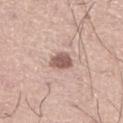<record>
<biopsy_status>not biopsied; imaged during a skin examination</biopsy_status>
<automated_metrics>
  <area_mm2_approx>5.0</area_mm2_approx>
  <eccentricity>0.45</eccentricity>
  <shape_asymmetry>0.25</shape_asymmetry>
  <cielab_L>57</cielab_L>
  <cielab_a>19</cielab_a>
  <cielab_b>24</cielab_b>
  <vs_skin_darker_L>14.0</vs_skin_darker_L>
  <vs_skin_contrast_norm>9.0</vs_skin_contrast_norm>
</automated_metrics>
<site>leg</site>
<image>
  <source>total-body photography crop</source>
  <field_of_view_mm>15</field_of_view_mm>
</image>
<patient>
  <sex>male</sex>
  <age_approx>35</age_approx>
</patient>
<lighting>white-light</lighting>
</record>